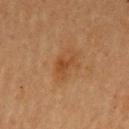Imaged during a routine full-body skin examination; the lesion was not biopsied and no histopathology is available.
The tile uses cross-polarized illumination.
The lesion's longest dimension is about 2.5 mm.
A lesion tile, about 15 mm wide, cut from a 3D total-body photograph.
The lesion is located on the left upper arm.
The total-body-photography lesion software estimated a lesion area of about 3 mm², an eccentricity of roughly 0.8, and two-axis asymmetry of about 0.45. The analysis additionally found roughly 7 lightness units darker than nearby skin and a normalized lesion–skin contrast near 6. It also reported an automated nevus-likeness rating near 5 out of 100 and lesion-presence confidence of about 100/100.
A female patient, aged approximately 60.